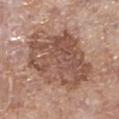Captured during whole-body skin photography for melanoma surveillance; the lesion was not biopsied.
The recorded lesion diameter is about 8.5 mm.
An algorithmic analysis of the crop reported a classifier nevus-likeness of about 35/100 and a lesion-detection confidence of about 100/100.
A 15 mm crop from a total-body photograph taken for skin-cancer surveillance.
From the leg.
Captured under white-light illumination.
A female patient aged 73–77.